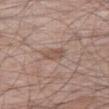This lesion was catalogued during total-body skin photography and was not selected for biopsy. On the right thigh. A male patient in their 60s. This is a white-light tile. A region of skin cropped from a whole-body photographic capture, roughly 15 mm wide. Longest diameter approximately 2.5 mm.